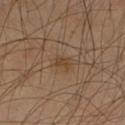Context: The tile uses cross-polarized illumination. A male subject, in their mid- to late 50s. Measured at roughly 2 mm in maximum diameter. A roughly 15 mm field-of-view crop from a total-body skin photograph. The lesion is located on the left upper arm.